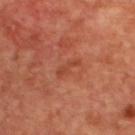biopsy_status: not biopsied; imaged during a skin examination
image:
  source: total-body photography crop
  field_of_view_mm: 15
site: upper back
lesion_size:
  long_diameter_mm_approx: 3.0
lighting: cross-polarized
patient:
  sex: female
  age_approx: 45